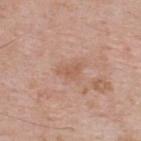Recorded during total-body skin imaging; not selected for excision or biopsy.
The lesion is on the back.
A 15 mm crop from a total-body photograph taken for skin-cancer surveillance.
A male patient approximately 60 years of age.
The total-body-photography lesion software estimated a lesion–skin lightness drop of about 7 and a normalized lesion–skin contrast near 5.5.
The tile uses white-light illumination.